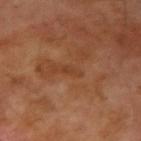follow-up: imaged on a skin check; not biopsied
tile lighting: cross-polarized illumination
patient: male, aged 68 to 72
image source: 15 mm crop, total-body photography
image-analysis metrics: a footprint of about 2 mm², an outline eccentricity of about 0.95 (0 = round, 1 = elongated), and a shape-asymmetry score of about 0.45 (0 = symmetric); a mean CIELAB color near L≈39 a*≈23 b*≈34 and a lesion–skin lightness drop of about 6; border irregularity of about 5 on a 0–10 scale, a color-variation rating of about 0/10, and radial color variation of about 0; lesion-presence confidence of about 85/100
anatomic site: the right upper arm
diameter: ≈2.5 mm